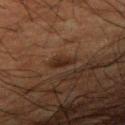| feature | finding |
|---|---|
| notes | imaged on a skin check; not biopsied |
| lesion size | about 3 mm |
| patient | male, approximately 60 years of age |
| site | the right upper arm |
| image | ~15 mm crop, total-body skin-cancer survey |
| tile lighting | cross-polarized illumination |